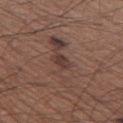| feature | finding |
|---|---|
| image-analysis metrics | an outline eccentricity of about 0.8 (0 = round, 1 = elongated); a border-irregularity rating of about 2/10, internal color variation of about 1.5 on a 0–10 scale, and peripheral color asymmetry of about 0.5; a classifier nevus-likeness of about 0/100 |
| patient | male, aged approximately 65 |
| lighting | white-light |
| body site | the left thigh |
| diameter | about 2.5 mm |
| acquisition | total-body-photography crop, ~15 mm field of view |